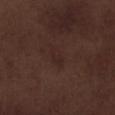{
  "biopsy_status": "not biopsied; imaged during a skin examination",
  "site": "leg",
  "image": {
    "source": "total-body photography crop",
    "field_of_view_mm": 15
  },
  "patient": {
    "sex": "male",
    "age_approx": 70
  },
  "lesion_size": {
    "long_diameter_mm_approx": 3.0
  }
}